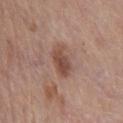This lesion was catalogued during total-body skin photography and was not selected for biopsy.
The total-body-photography lesion software estimated a lesion area of about 7 mm², an eccentricity of roughly 0.8, and a symmetry-axis asymmetry near 0.25. The software also gave roughly 10 lightness units darker than nearby skin and a normalized border contrast of about 8. The analysis additionally found border irregularity of about 3 on a 0–10 scale, a color-variation rating of about 3.5/10, and a peripheral color-asymmetry measure near 1. The analysis additionally found a classifier nevus-likeness of about 80/100 and a detector confidence of about 100 out of 100 that the crop contains a lesion.
Measured at roughly 3.5 mm in maximum diameter.
Cropped from a whole-body photographic skin survey; the tile spans about 15 mm.
Captured under white-light illumination.
A male patient, aged around 65.
Located on the chest.